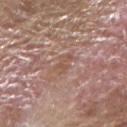Impression: No biopsy was performed on this lesion — it was imaged during a full skin examination and was not determined to be concerning. Context: A 15 mm crop from a total-body photograph taken for skin-cancer surveillance. The lesion is located on the right forearm. A male patient, about 65 years old. Captured under white-light illumination. The recorded lesion diameter is about 2.5 mm.